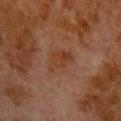<tbp_lesion>
<biopsy_status>not biopsied; imaged during a skin examination</biopsy_status>
<lighting>cross-polarized</lighting>
<patient>
  <sex>male</sex>
  <age_approx>80</age_approx>
</patient>
<automated_metrics>
  <shape_asymmetry>0.35</shape_asymmetry>
  <nevus_likeness_0_100>0</nevus_likeness_0_100>
  <lesion_detection_confidence_0_100>100</lesion_detection_confidence_0_100>
</automated_metrics>
<image>
  <source>total-body photography crop</source>
  <field_of_view_mm>15</field_of_view_mm>
</image>
<site>chest</site>
<lesion_size>
  <long_diameter_mm_approx>4.0</long_diameter_mm_approx>
</lesion_size>
</tbp_lesion>